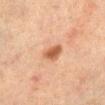Captured during whole-body skin photography for melanoma surveillance; the lesion was not biopsied.
A female patient, in their mid- to late 50s.
On the leg.
A 15 mm close-up tile from a total-body photography series done for melanoma screening.